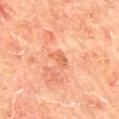Findings:
- biopsy status: imaged on a skin check; not biopsied
- site: the mid back
- subject: male, approximately 65 years of age
- acquisition: total-body-photography crop, ~15 mm field of view